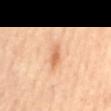follow-up: no biopsy performed (imaged during a skin exam).